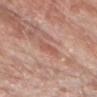Part of a total-body skin-imaging series; this lesion was reviewed on a skin check and was not flagged for biopsy.